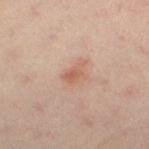biopsy status: imaged on a skin check; not biopsied
image: ~15 mm crop, total-body skin-cancer survey
lighting: cross-polarized
lesion diameter: about 2.5 mm
anatomic site: the right leg
subject: female, aged approximately 40